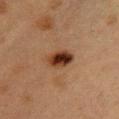No biopsy was performed on this lesion — it was imaged during a full skin examination and was not determined to be concerning. A 15 mm close-up extracted from a 3D total-body photography capture. Approximately 3.5 mm at its widest. A female subject, approximately 40 years of age. Captured under cross-polarized illumination. Located on the chest.